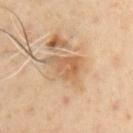Q: Was a biopsy performed?
A: imaged on a skin check; not biopsied
Q: What is the anatomic site?
A: the chest
Q: What did automated image analysis measure?
A: an area of roughly 9.5 mm² and two-axis asymmetry of about 0.45; a lesion color around L≈58 a*≈18 b*≈34 in CIELAB, about 9 CIELAB-L* units darker than the surrounding skin, and a normalized lesion–skin contrast near 6.5; border irregularity of about 5 on a 0–10 scale, a within-lesion color-variation index near 4.5/10, and peripheral color asymmetry of about 1.5; a classifier nevus-likeness of about 0/100 and lesion-presence confidence of about 100/100
Q: What lighting was used for the tile?
A: cross-polarized illumination
Q: What kind of image is this?
A: ~15 mm crop, total-body skin-cancer survey
Q: What are the patient's age and sex?
A: male, approximately 50 years of age
Q: How large is the lesion?
A: about 5 mm